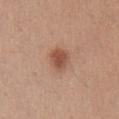site — the chest
lighting — white-light
TBP lesion metrics — an average lesion color of about L≈51 a*≈23 b*≈30 (CIELAB), roughly 11 lightness units darker than nearby skin, and a normalized lesion–skin contrast near 8
subject — female, aged 23 to 27
image source — ~15 mm tile from a whole-body skin photo